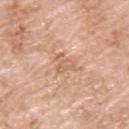Case summary:
– workup: imaged on a skin check; not biopsied
– site: the upper back
– diameter: about 2.5 mm
– tile lighting: white-light
– acquisition: 15 mm crop, total-body photography
– subject: male, about 60 years old
– TBP lesion metrics: a mean CIELAB color near L≈63 a*≈21 b*≈34 and about 7 CIELAB-L* units darker than the surrounding skin